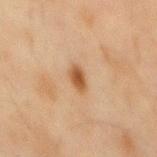  image:
    source: total-body photography crop
    field_of_view_mm: 15
  automated_metrics:
    area_mm2_approx: 4.0
    shape_asymmetry: 0.35
    cielab_L: 44
    cielab_a: 18
    cielab_b: 32
    vs_skin_darker_L: 11.0
    vs_skin_contrast_norm: 9.0
    lesion_detection_confidence_0_100: 100
  patient:
    sex: male
    age_approx: 45
  lesion_size:
    long_diameter_mm_approx: 3.0
  site: mid back
  lighting: cross-polarized